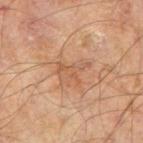Automated tile analysis of the lesion measured a color-variation rating of about 1/10 and a peripheral color-asymmetry measure near 0.5. And it measured a nevus-likeness score of about 0/100 and a lesion-detection confidence of about 100/100. The subject is a male roughly 65 years of age. From the arm. The lesion's longest dimension is about 4 mm. A close-up tile cropped from a whole-body skin photograph, about 15 mm across.